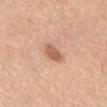A female patient, aged around 65. A region of skin cropped from a whole-body photographic capture, roughly 15 mm wide. The lesion is on the mid back. Imaged with white-light lighting.